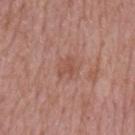Notes:
• notes: no biopsy performed (imaged during a skin exam)
• illumination: white-light
• automated metrics: a lesion area of about 3 mm², an outline eccentricity of about 0.75 (0 = round, 1 = elongated), and a symmetry-axis asymmetry near 0.45; an average lesion color of about L≈51 a*≈24 b*≈28 (CIELAB) and a lesion-to-skin contrast of about 5.5 (normalized; higher = more distinct); border irregularity of about 4.5 on a 0–10 scale, internal color variation of about 1 on a 0–10 scale, and peripheral color asymmetry of about 0.5
• subject: male, aged 63 to 67
• body site: the mid back
• image source: 15 mm crop, total-body photography